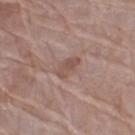Assessment: Captured during whole-body skin photography for melanoma surveillance; the lesion was not biopsied. Clinical summary: A female subject approximately 75 years of age. Longest diameter approximately 3 mm. The lesion-visualizer software estimated a footprint of about 4.5 mm², an eccentricity of roughly 0.85, and two-axis asymmetry of about 0.25. It also reported a mean CIELAB color near L≈51 a*≈18 b*≈24 and about 8 CIELAB-L* units darker than the surrounding skin. And it measured a border-irregularity rating of about 2.5/10 and radial color variation of about 1. Located on the right lower leg. A roughly 15 mm field-of-view crop from a total-body skin photograph. Imaged with white-light lighting.